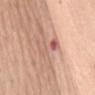Q: Was this lesion biopsied?
A: imaged on a skin check; not biopsied
Q: Who is the patient?
A: female, roughly 70 years of age
Q: Lesion location?
A: the mid back
Q: What did automated image analysis measure?
A: a lesion area of about 6.5 mm², a shape eccentricity near 0.9, and two-axis asymmetry of about 0.45
Q: How large is the lesion?
A: ~4.5 mm (longest diameter)
Q: How was the tile lit?
A: white-light
Q: What kind of image is this?
A: 15 mm crop, total-body photography